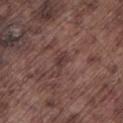notes — imaged on a skin check; not biopsied | location — the left lower leg | subject — male, in their mid- to late 70s | image source — ~15 mm tile from a whole-body skin photo | TBP lesion metrics — a lesion area of about 3.5 mm², an outline eccentricity of about 0.85 (0 = round, 1 = elongated), and a shape-asymmetry score of about 0.4 (0 = symmetric); a border-irregularity rating of about 4/10, a color-variation rating of about 2/10, and peripheral color asymmetry of about 0.5.